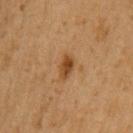| key | value |
|---|---|
| size | ≈3 mm |
| anatomic site | the left upper arm |
| illumination | cross-polarized |
| patient | male, aged 53–57 |
| acquisition | ~15 mm crop, total-body skin-cancer survey |